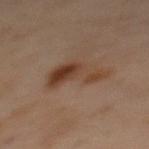follow-up = no biopsy performed (imaged during a skin exam)
tile lighting = cross-polarized illumination
diameter = ≈6 mm
image-analysis metrics = a border-irregularity rating of about 6/10, a color-variation rating of about 5/10, and peripheral color asymmetry of about 1.5; a nevus-likeness score of about 75/100 and a lesion-detection confidence of about 100/100
body site = the back
acquisition = total-body-photography crop, ~15 mm field of view
subject = female, aged approximately 55